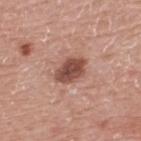Assessment: This lesion was catalogued during total-body skin photography and was not selected for biopsy. Acquisition and patient details: The subject is a male aged 73–77. A 15 mm close-up extracted from a 3D total-body photography capture. Longest diameter approximately 4 mm. The lesion is located on the upper back. The lesion-visualizer software estimated a lesion–skin lightness drop of about 15 and a normalized lesion–skin contrast near 10. It also reported an automated nevus-likeness rating near 75 out of 100 and a detector confidence of about 100 out of 100 that the crop contains a lesion. The tile uses white-light illumination.